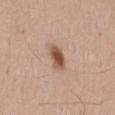The lesion was photographed on a routine skin check and not biopsied; there is no pathology result. A 15 mm close-up extracted from a 3D total-body photography capture. The lesion is located on the abdomen. A male patient in their mid-50s.